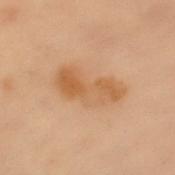No biopsy was performed on this lesion — it was imaged during a full skin examination and was not determined to be concerning.
A 15 mm close-up extracted from a 3D total-body photography capture.
From the upper back.
The tile uses cross-polarized illumination.
The subject is a female in their 60s.